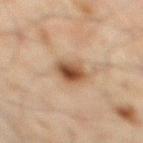biopsy status — total-body-photography surveillance lesion; no biopsy
location — the right lower leg
acquisition — 15 mm crop, total-body photography
size — ≈4 mm
subject — male, about 45 years old
illumination — cross-polarized
automated lesion analysis — a mean CIELAB color near L≈44 a*≈17 b*≈29, a lesion–skin lightness drop of about 13, and a normalized border contrast of about 10; a peripheral color-asymmetry measure near 2.5; an automated nevus-likeness rating near 95 out of 100